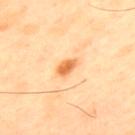No biopsy was performed on this lesion — it was imaged during a full skin examination and was not determined to be concerning.
A male patient aged around 45.
The total-body-photography lesion software estimated an average lesion color of about L≈67 a*≈27 b*≈46 (CIELAB) and roughly 13 lightness units darker than nearby skin. The software also gave internal color variation of about 2 on a 0–10 scale and peripheral color asymmetry of about 0.5.
Imaged with cross-polarized lighting.
On the upper back.
The lesion's longest dimension is about 2.5 mm.
Cropped from a total-body skin-imaging series; the visible field is about 15 mm.